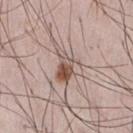Clinical impression: Imaged during a routine full-body skin examination; the lesion was not biopsied and no histopathology is available. Clinical summary: The subject is a male aged 33 to 37. Cropped from a whole-body photographic skin survey; the tile spans about 15 mm. The lesion is located on the chest.